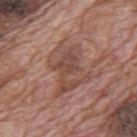<lesion>
<biopsy_status>not biopsied; imaged during a skin examination</biopsy_status>
<site>mid back</site>
<image>
  <source>total-body photography crop</source>
  <field_of_view_mm>15</field_of_view_mm>
</image>
<patient>
  <sex>male</sex>
  <age_approx>70</age_approx>
</patient>
</lesion>